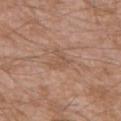• image: 15 mm crop, total-body photography
• location: the back
• patient: male, roughly 60 years of age